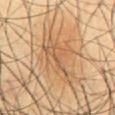lighting: cross-polarized
image:
  source: total-body photography crop
  field_of_view_mm: 15
lesion_size:
  long_diameter_mm_approx: 5.5
patient:
  sex: male
  age_approx: 60
automated_metrics:
  border_irregularity_0_10: 7.5
  color_variation_0_10: 4.5
site: abdomen